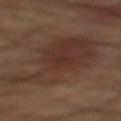Q: Is there a histopathology result?
A: total-body-photography surveillance lesion; no biopsy
Q: What lighting was used for the tile?
A: cross-polarized
Q: How was this image acquired?
A: ~15 mm crop, total-body skin-cancer survey
Q: What are the patient's age and sex?
A: male, roughly 65 years of age
Q: What is the anatomic site?
A: the mid back